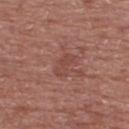| field | value |
|---|---|
| lesion diameter | ~3.5 mm (longest diameter) |
| location | the upper back |
| subject | male, about 55 years old |
| acquisition | ~15 mm crop, total-body skin-cancer survey |
| illumination | white-light illumination |